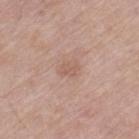Clinical summary:
A male subject roughly 85 years of age or older. Longest diameter approximately 2.5 mm. An algorithmic analysis of the crop reported an area of roughly 3.5 mm², a shape eccentricity near 0.7, and two-axis asymmetry of about 0.3. It also reported internal color variation of about 1 on a 0–10 scale and peripheral color asymmetry of about 0.5. Located on the left thigh. This is a white-light tile. This image is a 15 mm lesion crop taken from a total-body photograph.